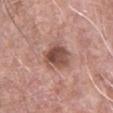Captured during whole-body skin photography for melanoma surveillance; the lesion was not biopsied.
Approximately 4 mm at its widest.
A male patient aged 48–52.
The tile uses white-light illumination.
Cropped from a whole-body photographic skin survey; the tile spans about 15 mm.
Located on the chest.